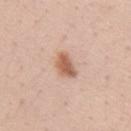workup: no biopsy performed (imaged during a skin exam)
image-analysis metrics: a lesion area of about 5.5 mm², a shape eccentricity near 0.8, and two-axis asymmetry of about 0.2; border irregularity of about 2 on a 0–10 scale, internal color variation of about 3 on a 0–10 scale, and radial color variation of about 1
anatomic site: the right upper arm
image source: total-body-photography crop, ~15 mm field of view
lesion size: ≈3.5 mm
lighting: white-light illumination
subject: female, aged around 40